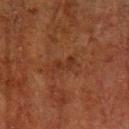{"biopsy_status": "not biopsied; imaged during a skin examination", "image": {"source": "total-body photography crop", "field_of_view_mm": 15}, "site": "left lower leg", "patient": {"sex": "male", "age_approx": 80}}